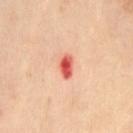Context:
This image is a 15 mm lesion crop taken from a total-body photograph. Captured under cross-polarized illumination. The total-body-photography lesion software estimated a footprint of about 4.5 mm², an outline eccentricity of about 0.7 (0 = round, 1 = elongated), and two-axis asymmetry of about 0.15. The software also gave a nevus-likeness score of about 0/100 and lesion-presence confidence of about 100/100. The subject is a female roughly 55 years of age. On the left thigh. Approximately 2.5 mm at its widest.